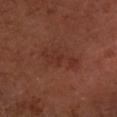The lesion was tiled from a total-body skin photograph and was not biopsied. A 15 mm close-up tile from a total-body photography series done for melanoma screening. A male subject, about 55 years old. An algorithmic analysis of the crop reported a lesion area of about 7.5 mm², a shape eccentricity near 0.9, and a symmetry-axis asymmetry near 0.45. The software also gave an average lesion color of about L≈32 a*≈24 b*≈26 (CIELAB), roughly 5 lightness units darker than nearby skin, and a lesion-to-skin contrast of about 5 (normalized; higher = more distinct). The analysis additionally found internal color variation of about 2 on a 0–10 scale and radial color variation of about 0.5. The analysis additionally found a nevus-likeness score of about 0/100 and a lesion-detection confidence of about 100/100. This is a cross-polarized tile. Located on the right forearm.